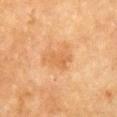The lesion was photographed on a routine skin check and not biopsied; there is no pathology result. The tile uses cross-polarized illumination. From the chest. A 15 mm close-up extracted from a 3D total-body photography capture. The patient is a female aged approximately 55.